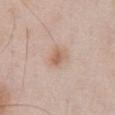workup: total-body-photography surveillance lesion; no biopsy | diameter: ≈2.5 mm | anatomic site: the abdomen | automated metrics: an average lesion color of about L≈61 a*≈18 b*≈29 (CIELAB), a lesion–skin lightness drop of about 9, and a normalized lesion–skin contrast near 6.5; a classifier nevus-likeness of about 85/100 and a lesion-detection confidence of about 100/100 | image: ~15 mm crop, total-body skin-cancer survey | illumination: white-light illumination | patient: male, aged 53 to 57.